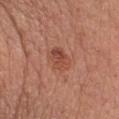| field | value |
|---|---|
| location | the chest |
| lesion diameter | about 3.5 mm |
| image-analysis metrics | an area of roughly 7 mm² and an eccentricity of roughly 0.7; a border-irregularity index near 1.5/10, a color-variation rating of about 6/10, and radial color variation of about 2 |
| lighting | white-light |
| image | total-body-photography crop, ~15 mm field of view |
| subject | female, in their mid-50s |